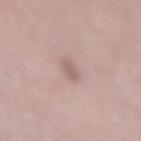Notes:
– workup — no biopsy performed (imaged during a skin exam)